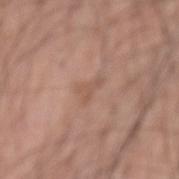Impression:
This lesion was catalogued during total-body skin photography and was not selected for biopsy.
Image and clinical context:
Measured at roughly 3 mm in maximum diameter. The total-body-photography lesion software estimated an area of roughly 3.5 mm², an eccentricity of roughly 0.85, and a shape-asymmetry score of about 0.55 (0 = symmetric). It also reported lesion-presence confidence of about 95/100. A 15 mm close-up extracted from a 3D total-body photography capture. A male subject, in their 60s. The lesion is located on the left forearm.